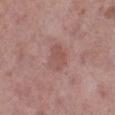image source=~15 mm tile from a whole-body skin photo | subject=female, approximately 50 years of age | body site=the right lower leg.